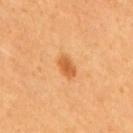  biopsy_status: not biopsied; imaged during a skin examination
  image:
    source: total-body photography crop
    field_of_view_mm: 15
  patient:
    sex: male
    age_approx: 60
  site: back